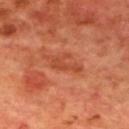Q: Is there a histopathology result?
A: total-body-photography surveillance lesion; no biopsy
Q: Lesion size?
A: about 4 mm
Q: What lighting was used for the tile?
A: cross-polarized
Q: What kind of image is this?
A: total-body-photography crop, ~15 mm field of view
Q: What did automated image analysis measure?
A: a footprint of about 5.5 mm², an eccentricity of roughly 0.9, and a symmetry-axis asymmetry near 0.45; a border-irregularity index near 6/10, a within-lesion color-variation index near 0.5/10, and radial color variation of about 0; lesion-presence confidence of about 100/100
Q: What are the patient's age and sex?
A: male, approximately 70 years of age
Q: What is the anatomic site?
A: the mid back